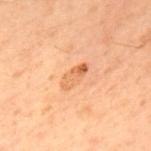biopsy_status: not biopsied; imaged during a skin examination
patient:
  sex: male
  age_approx: 60
lighting: cross-polarized
lesion_size:
  long_diameter_mm_approx: 4.0
automated_metrics:
  border_irregularity_0_10: 2.5
  color_variation_0_10: 7.0
  peripheral_color_asymmetry: 2.5
image:
  source: total-body photography crop
  field_of_view_mm: 15
site: upper back